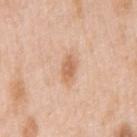<lesion>
  <biopsy_status>not biopsied; imaged during a skin examination</biopsy_status>
  <patient>
    <sex>female</sex>
    <age_approx>45</age_approx>
  </patient>
  <automated_metrics>
    <cielab_L>64</cielab_L>
    <cielab_a>22</cielab_a>
    <cielab_b>35</cielab_b>
    <vs_skin_contrast_norm>7.0</vs_skin_contrast_norm>
    <nevus_likeness_0_100>60</nevus_likeness_0_100>
    <lesion_detection_confidence_0_100>100</lesion_detection_confidence_0_100>
  </automated_metrics>
  <lighting>white-light</lighting>
  <lesion_size>
    <long_diameter_mm_approx>3.0</long_diameter_mm_approx>
  </lesion_size>
  <image>
    <source>total-body photography crop</source>
    <field_of_view_mm>15</field_of_view_mm>
  </image>
  <site>right upper arm</site>
</lesion>